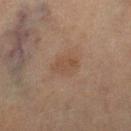Clinical impression:
Imaged during a routine full-body skin examination; the lesion was not biopsied and no histopathology is available.
Clinical summary:
Captured under cross-polarized illumination. A female subject, in their 70s. Automated tile analysis of the lesion measured a lesion area of about 6 mm², an outline eccentricity of about 0.75 (0 = round, 1 = elongated), and two-axis asymmetry of about 0.2. It also reported a classifier nevus-likeness of about 5/100 and lesion-presence confidence of about 100/100. The lesion is on the right lower leg. A 15 mm close-up extracted from a 3D total-body photography capture. Approximately 3.5 mm at its widest.